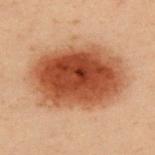Assessment:
Imaged during a routine full-body skin examination; the lesion was not biopsied and no histopathology is available.
Clinical summary:
This image is a 15 mm lesion crop taken from a total-body photograph. Automated image analysis of the tile measured a footprint of about 55 mm² and an eccentricity of roughly 0.75. The software also gave an average lesion color of about L≈42 a*≈22 b*≈31 (CIELAB), about 15 CIELAB-L* units darker than the surrounding skin, and a normalized border contrast of about 11.5. It also reported a border-irregularity rating of about 2/10, a within-lesion color-variation index near 8/10, and radial color variation of about 2.5. And it measured lesion-presence confidence of about 100/100. The lesion is located on the upper back. The subject is a male aged approximately 55. Longest diameter approximately 10.5 mm. Imaged with cross-polarized lighting.